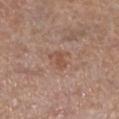Imaged during a routine full-body skin examination; the lesion was not biopsied and no histopathology is available. Approximately 2.5 mm at its widest. From the left lower leg. The subject is a female aged 53 to 57. Captured under white-light illumination. A 15 mm crop from a total-body photograph taken for skin-cancer surveillance. Automated tile analysis of the lesion measured an average lesion color of about L≈51 a*≈20 b*≈29 (CIELAB), about 7 CIELAB-L* units darker than the surrounding skin, and a normalized border contrast of about 6. And it measured an automated nevus-likeness rating near 0 out of 100 and lesion-presence confidence of about 100/100.